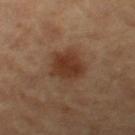Clinical impression: No biopsy was performed on this lesion — it was imaged during a full skin examination and was not determined to be concerning. Image and clinical context: This image is a 15 mm lesion crop taken from a total-body photograph. The lesion-visualizer software estimated an eccentricity of roughly 0.6 and a shape-asymmetry score of about 0.25 (0 = symmetric). The software also gave a lesion color around L≈37 a*≈20 b*≈30 in CIELAB, roughly 9 lightness units darker than nearby skin, and a normalized border contrast of about 8.5. And it measured a nevus-likeness score of about 95/100 and a lesion-detection confidence of about 100/100. Measured at roughly 5 mm in maximum diameter. From the left thigh. Imaged with cross-polarized lighting. A female subject about 60 years old.